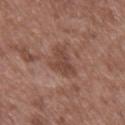workup: catalogued during a skin exam; not biopsied
location: the mid back
patient: male, aged 48 to 52
diameter: ≈4.5 mm
TBP lesion metrics: an eccentricity of roughly 0.8 and a shape-asymmetry score of about 0.45 (0 = symmetric); border irregularity of about 4.5 on a 0–10 scale and a within-lesion color-variation index near 2/10; a nevus-likeness score of about 0/100 and a lesion-detection confidence of about 100/100
illumination: white-light illumination
acquisition: ~15 mm crop, total-body skin-cancer survey